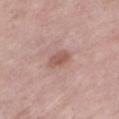Automated tile analysis of the lesion measured an outline eccentricity of about 0.85 (0 = round, 1 = elongated) and two-axis asymmetry of about 0.2. The analysis additionally found a nevus-likeness score of about 15/100 and lesion-presence confidence of about 100/100. A female subject roughly 70 years of age. Located on the left lower leg. Captured under white-light illumination. A 15 mm crop from a total-body photograph taken for skin-cancer surveillance.